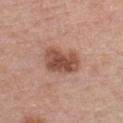Impression:
Part of a total-body skin-imaging series; this lesion was reviewed on a skin check and was not flagged for biopsy.
Image and clinical context:
A male subject roughly 60 years of age. Longest diameter approximately 5 mm. Captured under white-light illumination. A 15 mm close-up tile from a total-body photography series done for melanoma screening. Automated tile analysis of the lesion measured a border-irregularity rating of about 2/10. The analysis additionally found an automated nevus-likeness rating near 80 out of 100. The lesion is on the chest.